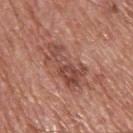| field | value |
|---|---|
| notes | catalogued during a skin exam; not biopsied |
| lesion diameter | ≈6 mm |
| patient | male, about 65 years old |
| imaging modality | total-body-photography crop, ~15 mm field of view |
| body site | the upper back |
| illumination | white-light illumination |